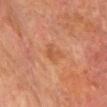tile lighting: cross-polarized
image-analysis metrics: a mean CIELAB color near L≈53 a*≈25 b*≈37 and about 6 CIELAB-L* units darker than the surrounding skin; border irregularity of about 4 on a 0–10 scale, internal color variation of about 2 on a 0–10 scale, and a peripheral color-asymmetry measure near 0.5; a classifier nevus-likeness of about 0/100 and lesion-presence confidence of about 100/100
lesion size: ≈3 mm
site: the head or neck
image source: ~15 mm tile from a whole-body skin photo
patient: male, aged around 65